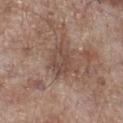  biopsy_status: not biopsied; imaged during a skin examination
  site: right lower leg
  patient:
    sex: male
    age_approx: 70
  image:
    source: total-body photography crop
    field_of_view_mm: 15